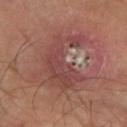notes=no biopsy performed (imaged during a skin exam)
image source=total-body-photography crop, ~15 mm field of view
subject=male, aged approximately 65
site=the left lower leg